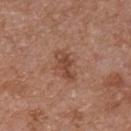  patient:
    sex: male
    age_approx: 55
  lighting: white-light
  image:
    source: total-body photography crop
    field_of_view_mm: 15
  site: chest
  lesion_size:
    long_diameter_mm_approx: 4.0